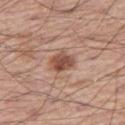Captured during whole-body skin photography for melanoma surveillance; the lesion was not biopsied.
A 15 mm close-up tile from a total-body photography series done for melanoma screening.
Captured under white-light illumination.
A male subject approximately 60 years of age.
From the right thigh.